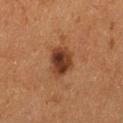Assessment: Recorded during total-body skin imaging; not selected for excision or biopsy. Acquisition and patient details: The lesion is located on the left thigh. Approximately 3.5 mm at its widest. The subject is a female about 50 years old. Captured under cross-polarized illumination. A 15 mm crop from a total-body photograph taken for skin-cancer surveillance.